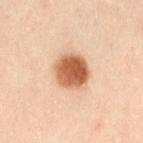No biopsy was performed on this lesion — it was imaged during a full skin examination and was not determined to be concerning.
The recorded lesion diameter is about 4 mm.
A female subject about 30 years old.
Imaged with cross-polarized lighting.
From the front of the torso.
A lesion tile, about 15 mm wide, cut from a 3D total-body photograph.